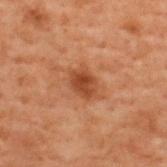notes: no biopsy performed (imaged during a skin exam)
patient: male, aged 68 to 72
imaging modality: total-body-photography crop, ~15 mm field of view
diameter: ~3.5 mm (longest diameter)
automated metrics: a footprint of about 6.5 mm², an eccentricity of roughly 0.7, and two-axis asymmetry of about 0.2; an average lesion color of about L≈35 a*≈21 b*≈30 (CIELAB) and a lesion-to-skin contrast of about 8 (normalized; higher = more distinct); a classifier nevus-likeness of about 70/100 and a lesion-detection confidence of about 100/100
illumination: cross-polarized illumination
location: the upper back